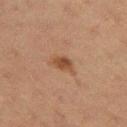notes=total-body-photography surveillance lesion; no biopsy
imaging modality=~15 mm tile from a whole-body skin photo
patient=female, approximately 20 years of age
anatomic site=the left thigh